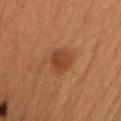Assessment: Part of a total-body skin-imaging series; this lesion was reviewed on a skin check and was not flagged for biopsy. Clinical summary: The tile uses cross-polarized illumination. The patient is a male roughly 50 years of age. Located on the chest. A close-up tile cropped from a whole-body skin photograph, about 15 mm across. Approximately 3 mm at its widest. The total-body-photography lesion software estimated an eccentricity of roughly 0.55 and two-axis asymmetry of about 0.2. And it measured internal color variation of about 2.5 on a 0–10 scale and a peripheral color-asymmetry measure near 1. The analysis additionally found a nevus-likeness score of about 80/100 and lesion-presence confidence of about 100/100.